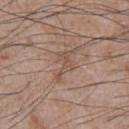Captured during whole-body skin photography for melanoma surveillance; the lesion was not biopsied.
This image is a 15 mm lesion crop taken from a total-body photograph.
Located on the chest.
Imaged with white-light lighting.
A male patient, aged 73 to 77.
Measured at roughly 4 mm in maximum diameter.
Automated tile analysis of the lesion measured an outline eccentricity of about 0.9 (0 = round, 1 = elongated) and a symmetry-axis asymmetry near 0.55. And it measured an average lesion color of about L≈51 a*≈17 b*≈25 (CIELAB), roughly 7 lightness units darker than nearby skin, and a lesion-to-skin contrast of about 5 (normalized; higher = more distinct). It also reported border irregularity of about 7.5 on a 0–10 scale, a within-lesion color-variation index near 0/10, and a peripheral color-asymmetry measure near 0.